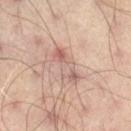{
  "biopsy_status": "not biopsied; imaged during a skin examination",
  "site": "right thigh",
  "automated_metrics": {
    "vs_skin_darker_L": 8.0,
    "border_irregularity_0_10": 4.5,
    "peripheral_color_asymmetry": 1.5
  },
  "lesion_size": {
    "long_diameter_mm_approx": 5.0
  },
  "image": {
    "source": "total-body photography crop",
    "field_of_view_mm": 15
  },
  "patient": {
    "sex": "male",
    "age_approx": 65
  },
  "lighting": "cross-polarized"
}